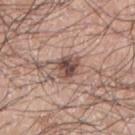biopsy status — total-body-photography surveillance lesion; no biopsy
image source — total-body-photography crop, ~15 mm field of view
patient — male, in their 70s
lesion diameter — about 3 mm
illumination — white-light illumination
anatomic site — the left arm
image-analysis metrics — a lesion color around L≈49 a*≈18 b*≈23 in CIELAB, roughly 14 lightness units darker than nearby skin, and a normalized border contrast of about 10; a nevus-likeness score of about 75/100 and a lesion-detection confidence of about 100/100